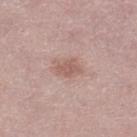acquisition — ~15 mm crop, total-body skin-cancer survey | size — ≈3.5 mm | illumination — white-light | anatomic site — the right lower leg | automated lesion analysis — a footprint of about 5.5 mm², a shape eccentricity near 0.75, and two-axis asymmetry of about 0.25; a classifier nevus-likeness of about 30/100 and a detector confidence of about 100 out of 100 that the crop contains a lesion | patient — female, approximately 45 years of age.